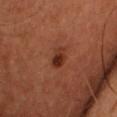- notes — imaged on a skin check; not biopsied
- size — about 3 mm
- acquisition — ~15 mm tile from a whole-body skin photo
- subject — male, aged 48–52
- anatomic site — the chest
- automated lesion analysis — a normalized lesion–skin contrast near 8.5; a border-irregularity rating of about 2.5/10 and radial color variation of about 1.5